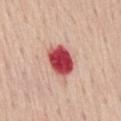Q: Is there a histopathology result?
A: no biopsy performed (imaged during a skin exam)
Q: Automated lesion metrics?
A: a lesion color around L≈51 a*≈39 b*≈26 in CIELAB, a lesion–skin lightness drop of about 22, and a lesion-to-skin contrast of about 13.5 (normalized; higher = more distinct)
Q: How was this image acquired?
A: ~15 mm crop, total-body skin-cancer survey
Q: Patient demographics?
A: male, roughly 60 years of age
Q: Lesion size?
A: about 4 mm
Q: How was the tile lit?
A: white-light illumination
Q: Lesion location?
A: the mid back